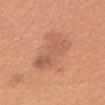Part of a total-body skin-imaging series; this lesion was reviewed on a skin check and was not flagged for biopsy.
A 15 mm close-up tile from a total-body photography series done for melanoma screening.
On the head or neck.
A female patient aged 23–27.